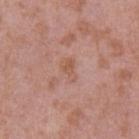{"biopsy_status": "not biopsied; imaged during a skin examination", "image": {"source": "total-body photography crop", "field_of_view_mm": 15}, "site": "left upper arm", "lighting": "white-light", "automated_metrics": {"area_mm2_approx": 3.5, "eccentricity": 0.85, "shape_asymmetry": 0.45, "border_irregularity_0_10": 4.0, "peripheral_color_asymmetry": 0.5, "nevus_likeness_0_100": 0, "lesion_detection_confidence_0_100": 100}, "lesion_size": {"long_diameter_mm_approx": 3.0}, "patient": {"sex": "male", "age_approx": 40}}